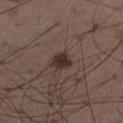Q: What kind of image is this?
A: total-body-photography crop, ~15 mm field of view
Q: Illumination type?
A: white-light illumination
Q: Lesion location?
A: the left thigh
Q: What is the lesion's diameter?
A: about 2.5 mm
Q: Who is the patient?
A: male, about 50 years old